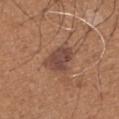Acquisition and patient details:
The lesion is on the left upper arm. The recorded lesion diameter is about 4 mm. The tile uses white-light illumination. Cropped from a total-body skin-imaging series; the visible field is about 15 mm. The lesion-visualizer software estimated an eccentricity of roughly 0.6. The analysis additionally found a lesion color around L≈45 a*≈20 b*≈26 in CIELAB and roughly 9 lightness units darker than nearby skin. A male patient, in their mid-60s.